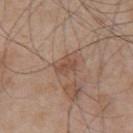The lesion was photographed on a routine skin check and not biopsied; there is no pathology result. A 15 mm crop from a total-body photograph taken for skin-cancer surveillance. A male patient, aged approximately 55. From the back. The lesion's longest dimension is about 2.5 mm. Imaged with white-light lighting. Automated image analysis of the tile measured a within-lesion color-variation index near 1/10 and peripheral color asymmetry of about 0.5. It also reported a nevus-likeness score of about 0/100 and lesion-presence confidence of about 100/100.